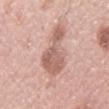notes: catalogued during a skin exam; not biopsied | TBP lesion metrics: a lesion area of about 13 mm², an outline eccentricity of about 0.85 (0 = round, 1 = elongated), and a symmetry-axis asymmetry near 0.65; roughly 12 lightness units darker than nearby skin and a normalized border contrast of about 7.5 | illumination: white-light | patient: female, roughly 50 years of age | body site: the left upper arm | image: ~15 mm tile from a whole-body skin photo | lesion diameter: about 6 mm.